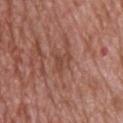biopsy status = catalogued during a skin exam; not biopsied | image-analysis metrics = an area of roughly 4 mm² and a shape eccentricity near 0.8; an automated nevus-likeness rating near 0 out of 100 and lesion-presence confidence of about 90/100 | lesion size = about 3 mm | subject = male, roughly 70 years of age | tile lighting = white-light | body site = the upper back | image source = ~15 mm crop, total-body skin-cancer survey.